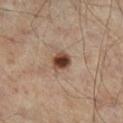workup: no biopsy performed (imaged during a skin exam)
lesion size: ≈3 mm
patient: male, aged approximately 65
image source: ~15 mm crop, total-body skin-cancer survey
automated lesion analysis: a lesion color around L≈42 a*≈18 b*≈26 in CIELAB and a normalized border contrast of about 11.5; a color-variation rating of about 6.5/10; lesion-presence confidence of about 100/100
site: the leg
tile lighting: cross-polarized